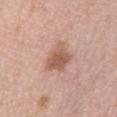follow-up = catalogued during a skin exam; not biopsied | lesion diameter = ≈4 mm | site = the left lower leg | image = 15 mm crop, total-body photography | patient = female, about 65 years old.